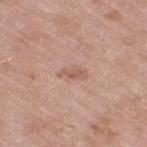biopsy_status: not biopsied; imaged during a skin examination
site: leg
image:
  source: total-body photography crop
  field_of_view_mm: 15
lesion_size:
  long_diameter_mm_approx: 3.0
lighting: white-light
patient:
  sex: female
  age_approx: 70